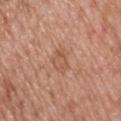Captured during whole-body skin photography for melanoma surveillance; the lesion was not biopsied. The total-body-photography lesion software estimated a footprint of about 5.5 mm², an eccentricity of roughly 0.65, and two-axis asymmetry of about 0.3. And it measured a nevus-likeness score of about 0/100 and lesion-presence confidence of about 100/100. A close-up tile cropped from a whole-body skin photograph, about 15 mm across. The recorded lesion diameter is about 3 mm. A male patient, approximately 50 years of age. The lesion is on the back.